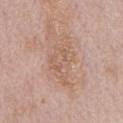{"site": "chest", "automated_metrics": {"area_mm2_approx": 10.0, "eccentricity": 0.85, "cielab_L": 60, "cielab_a": 19, "cielab_b": 28, "vs_skin_darker_L": 7.0, "border_irregularity_0_10": 7.0, "color_variation_0_10": 3.5, "peripheral_color_asymmetry": 1.0}, "patient": {"sex": "male", "age_approx": 70}, "lighting": "white-light", "image": {"source": "total-body photography crop", "field_of_view_mm": 15}}